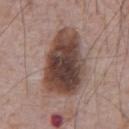Part of a total-body skin-imaging series; this lesion was reviewed on a skin check and was not flagged for biopsy. A male patient aged 68 to 72. Cropped from a whole-body photographic skin survey; the tile spans about 15 mm. The tile uses white-light illumination. From the front of the torso.